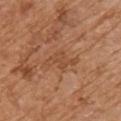| field | value |
|---|---|
| notes | no biopsy performed (imaged during a skin exam) |